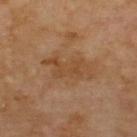{
  "biopsy_status": "not biopsied; imaged during a skin examination",
  "patient": {
    "sex": "male",
    "age_approx": 70
  },
  "image": {
    "source": "total-body photography crop",
    "field_of_view_mm": 15
  },
  "lighting": "cross-polarized",
  "site": "upper back",
  "lesion_size": {
    "long_diameter_mm_approx": 5.5
  }
}